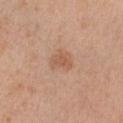The tile uses white-light illumination. A 15 mm close-up extracted from a 3D total-body photography capture. Automated image analysis of the tile measured an area of roughly 4.5 mm², an eccentricity of roughly 0.7, and a symmetry-axis asymmetry near 0.35. A male subject, aged approximately 75. From the arm. The recorded lesion diameter is about 3 mm.